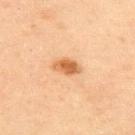notes: imaged on a skin check; not biopsied | subject: male, about 45 years old | illumination: cross-polarized illumination | body site: the upper back | automated lesion analysis: a lesion area of about 5 mm², a shape eccentricity near 0.8, and two-axis asymmetry of about 0.2; about 12 CIELAB-L* units darker than the surrounding skin and a lesion-to-skin contrast of about 8.5 (normalized; higher = more distinct) | image: total-body-photography crop, ~15 mm field of view.